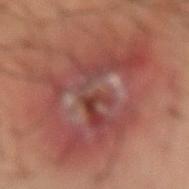biopsy status: imaged on a skin check; not biopsied | TBP lesion metrics: an area of roughly 65 mm², a shape eccentricity near 0.75, and two-axis asymmetry of about 0.25; a mean CIELAB color near L≈45 a*≈26 b*≈25, about 8 CIELAB-L* units darker than the surrounding skin, and a lesion-to-skin contrast of about 7 (normalized; higher = more distinct); a classifier nevus-likeness of about 0/100 and lesion-presence confidence of about 90/100 | site: the right forearm | patient: male, aged 43–47 | acquisition: 15 mm crop, total-body photography | size: about 11.5 mm.